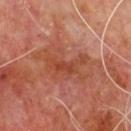The lesion is on the chest. The tile uses cross-polarized illumination. The subject is a male aged approximately 65. Longest diameter approximately 7 mm. A roughly 15 mm field-of-view crop from a total-body skin photograph.